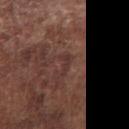Impression:
Imaged during a routine full-body skin examination; the lesion was not biopsied and no histopathology is available.
Clinical summary:
Located on the chest. The patient is a male about 75 years old. The recorded lesion diameter is about 3 mm. A lesion tile, about 15 mm wide, cut from a 3D total-body photograph. Automated image analysis of the tile measured an average lesion color of about L≈34 a*≈20 b*≈22 (CIELAB) and a lesion–skin lightness drop of about 5. And it measured border irregularity of about 5 on a 0–10 scale, a within-lesion color-variation index near 0/10, and peripheral color asymmetry of about 0. The analysis additionally found a nevus-likeness score of about 0/100.